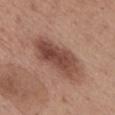Q: Was a biopsy performed?
A: catalogued during a skin exam; not biopsied
Q: Automated lesion metrics?
A: a lesion color around L≈46 a*≈22 b*≈26 in CIELAB and roughly 13 lightness units darker than nearby skin
Q: What is the imaging modality?
A: ~15 mm tile from a whole-body skin photo
Q: Illumination type?
A: white-light
Q: Who is the patient?
A: female, approximately 50 years of age
Q: How large is the lesion?
A: ≈7.5 mm
Q: What is the anatomic site?
A: the mid back